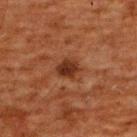biopsy status — catalogued during a skin exam; not biopsied
TBP lesion metrics — an area of roughly 5 mm², a shape eccentricity near 0.5, and a shape-asymmetry score of about 0.2 (0 = symmetric); an average lesion color of about L≈25 a*≈20 b*≈26 (CIELAB) and a lesion-to-skin contrast of about 10 (normalized; higher = more distinct); a nevus-likeness score of about 80/100
image — total-body-photography crop, ~15 mm field of view
subject — male, aged 58 to 62
body site — the upper back
illumination — cross-polarized